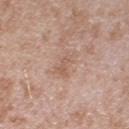Context: The lesion is on the left upper arm. A 15 mm close-up tile from a total-body photography series done for melanoma screening. Imaged with white-light lighting. Approximately 3.5 mm at its widest. A male patient, aged 43 to 47. The total-body-photography lesion software estimated a lesion area of about 5.5 mm² and a shape eccentricity near 0.9. And it measured a nevus-likeness score of about 0/100 and lesion-presence confidence of about 100/100.